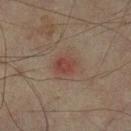<lesion>
  <biopsy_status>not biopsied; imaged during a skin examination</biopsy_status>
  <image>
    <source>total-body photography crop</source>
    <field_of_view_mm>15</field_of_view_mm>
  </image>
  <lighting>cross-polarized</lighting>
  <site>left lower leg</site>
  <patient>
    <sex>male</sex>
    <age_approx>75</age_approx>
  </patient>
</lesion>